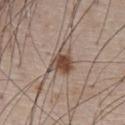Image and clinical context:
The patient is a male aged approximately 80. A roughly 15 mm field-of-view crop from a total-body skin photograph. Longest diameter approximately 3.5 mm. The lesion is on the front of the torso. Imaged with white-light lighting.